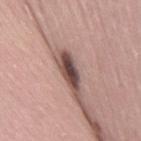Recorded during total-body skin imaging; not selected for excision or biopsy.
Cropped from a total-body skin-imaging series; the visible field is about 15 mm.
The lesion's longest dimension is about 4 mm.
The lesion is located on the right thigh.
A female patient, approximately 30 years of age.
Automated image analysis of the tile measured border irregularity of about 3.5 on a 0–10 scale. The analysis additionally found a nevus-likeness score of about 100/100 and lesion-presence confidence of about 80/100.